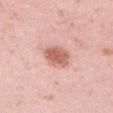  biopsy_status: not biopsied; imaged during a skin examination
  patient:
    sex: male
    age_approx: 35
  lesion_size:
    long_diameter_mm_approx: 3.5
  site: left upper arm
  image:
    source: total-body photography crop
    field_of_view_mm: 15
  lighting: white-light